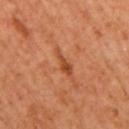Part of a total-body skin-imaging series; this lesion was reviewed on a skin check and was not flagged for biopsy.